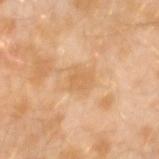biopsy status = imaged on a skin check; not biopsied